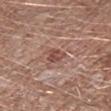Q: What lighting was used for the tile?
A: white-light illumination
Q: What did automated image analysis measure?
A: a mean CIELAB color near L≈48 a*≈22 b*≈25, about 10 CIELAB-L* units darker than the surrounding skin, and a normalized border contrast of about 7; a nevus-likeness score of about 0/100 and a detector confidence of about 100 out of 100 that the crop contains a lesion
Q: Where on the body is the lesion?
A: the right lower leg
Q: What is the lesion's diameter?
A: ≈2.5 mm
Q: How was this image acquired?
A: ~15 mm crop, total-body skin-cancer survey
Q: Who is the patient?
A: male, in their 60s